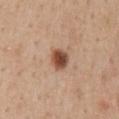{
  "image": {
    "source": "total-body photography crop",
    "field_of_view_mm": 15
  },
  "lesion_size": {
    "long_diameter_mm_approx": 2.5
  },
  "site": "mid back",
  "lighting": "white-light",
  "patient": {
    "sex": "male",
    "age_approx": 55
  }
}